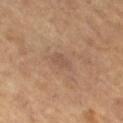{
  "biopsy_status": "not biopsied; imaged during a skin examination",
  "patient": {
    "sex": "female",
    "age_approx": 70
  },
  "image": {
    "source": "total-body photography crop",
    "field_of_view_mm": 15
  },
  "site": "leg",
  "lighting": "cross-polarized",
  "lesion_size": {
    "long_diameter_mm_approx": 2.5
  }
}